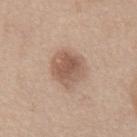biopsy_status: not biopsied; imaged during a skin examination
site: chest
image:
  source: total-body photography crop
  field_of_view_mm: 15
patient:
  sex: male
  age_approx: 60
lighting: white-light
automated_metrics:
  area_mm2_approx: 13.0
  eccentricity: 0.35
  shape_asymmetry: 0.15
  border_irregularity_0_10: 1.5
  peripheral_color_asymmetry: 1.5